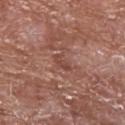Notes:
* notes · imaged on a skin check; not biopsied
* subject · male, roughly 65 years of age
* diameter · ~2.5 mm (longest diameter)
* location · the chest
* acquisition · ~15 mm tile from a whole-body skin photo
* automated metrics · an area of roughly 3 mm², an outline eccentricity of about 0.85 (0 = round, 1 = elongated), and two-axis asymmetry of about 0.4; a lesion color around L≈45 a*≈23 b*≈26 in CIELAB, roughly 7 lightness units darker than nearby skin, and a normalized border contrast of about 5.5; border irregularity of about 4 on a 0–10 scale, a within-lesion color-variation index near 0/10, and peripheral color asymmetry of about 0; a nevus-likeness score of about 0/100
* lighting · white-light illumination